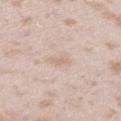biopsy status: imaged on a skin check; not biopsied
subject: female, in their mid- to late 20s
image: ~15 mm tile from a whole-body skin photo
anatomic site: the leg
automated lesion analysis: a footprint of about 2.5 mm², an outline eccentricity of about 0.9 (0 = round, 1 = elongated), and a shape-asymmetry score of about 0.3 (0 = symmetric); peripheral color asymmetry of about 0